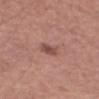Impression: The lesion was photographed on a routine skin check and not biopsied; there is no pathology result. Acquisition and patient details: On the left forearm. An algorithmic analysis of the crop reported an area of roughly 4 mm², an outline eccentricity of about 0.75 (0 = round, 1 = elongated), and a shape-asymmetry score of about 0.2 (0 = symmetric). The analysis additionally found a lesion color around L≈49 a*≈22 b*≈23 in CIELAB, about 10 CIELAB-L* units darker than the surrounding skin, and a normalized lesion–skin contrast near 7. And it measured a peripheral color-asymmetry measure near 0.5. And it measured an automated nevus-likeness rating near 65 out of 100 and a detector confidence of about 100 out of 100 that the crop contains a lesion. The patient is a male aged around 60. A roughly 15 mm field-of-view crop from a total-body skin photograph. The lesion's longest dimension is about 2.5 mm.